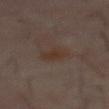Part of a total-body skin-imaging series; this lesion was reviewed on a skin check and was not flagged for biopsy.
The total-body-photography lesion software estimated a mean CIELAB color near L≈30 a*≈13 b*≈21 and roughly 4 lightness units darker than nearby skin. The software also gave border irregularity of about 2 on a 0–10 scale, internal color variation of about 2 on a 0–10 scale, and radial color variation of about 1.
Longest diameter approximately 3 mm.
A male patient, aged 58 to 62.
The lesion is on the abdomen.
Cropped from a total-body skin-imaging series; the visible field is about 15 mm.
Imaged with cross-polarized lighting.